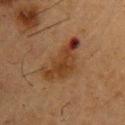Recorded during total-body skin imaging; not selected for excision or biopsy. A close-up tile cropped from a whole-body skin photograph, about 15 mm across. The patient is a male aged 53 to 57. This is a cross-polarized tile. Automated image analysis of the tile measured a shape eccentricity near 0.9 and two-axis asymmetry of about 0.5. And it measured a border-irregularity rating of about 6/10, internal color variation of about 5.5 on a 0–10 scale, and radial color variation of about 1.5. Approximately 6.5 mm at its widest. The lesion is on the upper back.